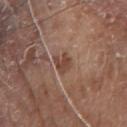{"biopsy_status": "not biopsied; imaged during a skin examination", "lesion_size": {"long_diameter_mm_approx": 3.0}, "site": "front of the torso", "image": {"source": "total-body photography crop", "field_of_view_mm": 15}, "automated_metrics": {"cielab_L": 44, "cielab_a": 20, "cielab_b": 27, "vs_skin_darker_L": 9.0, "vs_skin_contrast_norm": 7.0, "border_irregularity_0_10": 4.0, "color_variation_0_10": 2.0}, "patient": {"sex": "male", "age_approx": 80}, "lighting": "white-light"}